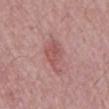  biopsy_status: not biopsied; imaged during a skin examination